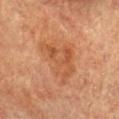Part of a total-body skin-imaging series; this lesion was reviewed on a skin check and was not flagged for biopsy. This is a cross-polarized tile. A 15 mm crop from a total-body photograph taken for skin-cancer surveillance. An algorithmic analysis of the crop reported a footprint of about 20 mm², an eccentricity of roughly 0.75, and two-axis asymmetry of about 0.4. The analysis additionally found a border-irregularity index near 4/10 and a within-lesion color-variation index near 4.5/10. A female subject, approximately 80 years of age. The lesion's longest dimension is about 6.5 mm. The lesion is located on the chest.